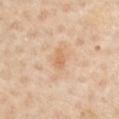{
  "biopsy_status": "not biopsied; imaged during a skin examination",
  "patient": {
    "sex": "male",
    "age_approx": 65
  },
  "lighting": "cross-polarized",
  "site": "chest",
  "lesion_size": {
    "long_diameter_mm_approx": 2.5
  },
  "automated_metrics": {
    "area_mm2_approx": 3.0,
    "eccentricity": 0.85,
    "shape_asymmetry": 0.3,
    "cielab_L": 65,
    "cielab_a": 21,
    "cielab_b": 36,
    "vs_skin_darker_L": 8.0,
    "vs_skin_contrast_norm": 5.5
  },
  "image": {
    "source": "total-body photography crop",
    "field_of_view_mm": 15
  }
}